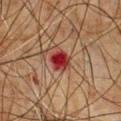{"biopsy_status": "not biopsied; imaged during a skin examination", "lighting": "cross-polarized", "patient": {"sex": "male", "age_approx": 50}, "lesion_size": {"long_diameter_mm_approx": 3.0}, "image": {"source": "total-body photography crop", "field_of_view_mm": 15}, "site": "chest", "automated_metrics": {"area_mm2_approx": 6.0, "eccentricity": 0.55, "shape_asymmetry": 0.25, "color_variation_0_10": 7.5, "peripheral_color_asymmetry": 2.5, "nevus_likeness_0_100": 0}}